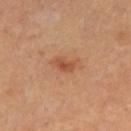Case summary:
• image · 15 mm crop, total-body photography
• subject · female, approximately 30 years of age
• anatomic site · the right thigh